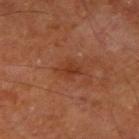The lesion is located on the left thigh. A 15 mm close-up tile from a total-body photography series done for melanoma screening. A male subject, aged 63 to 67. About 3 mm across.